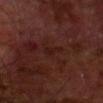workup=no biopsy performed (imaged during a skin exam) | image-analysis metrics=a lesion area of about 3 mm² and an eccentricity of roughly 0.9; a border-irregularity index near 4.5/10, internal color variation of about 1 on a 0–10 scale, and peripheral color asymmetry of about 0; an automated nevus-likeness rating near 0 out of 100 and a detector confidence of about 65 out of 100 that the crop contains a lesion | patient=male, about 70 years old | body site=the front of the torso | imaging modality=~15 mm crop, total-body skin-cancer survey | lesion size=≈3 mm.